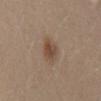The tile uses white-light illumination. Located on the mid back. The subject is a male roughly 30 years of age. About 3 mm across. This image is a 15 mm lesion crop taken from a total-body photograph.